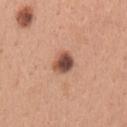notes: imaged on a skin check; not biopsied | site: the left upper arm | imaging modality: 15 mm crop, total-body photography | subject: female, aged around 30.